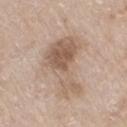| field | value |
|---|---|
| biopsy status | imaged on a skin check; not biopsied |
| image | ~15 mm crop, total-body skin-cancer survey |
| subject | female, aged approximately 75 |
| diameter | ~7.5 mm (longest diameter) |
| lighting | white-light illumination |
| body site | the leg |